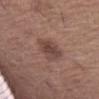image: 15 mm crop, total-body photography | anatomic site: the left thigh | patient: female, approximately 65 years of age | diameter: about 3.5 mm | automated lesion analysis: a lesion area of about 7.5 mm², an eccentricity of roughly 0.5, and a shape-asymmetry score of about 0.25 (0 = symmetric); a mean CIELAB color near L≈44 a*≈19 b*≈23, roughly 9 lightness units darker than nearby skin, and a normalized lesion–skin contrast near 7.5; border irregularity of about 2.5 on a 0–10 scale, a color-variation rating of about 3/10, and peripheral color asymmetry of about 1; lesion-presence confidence of about 100/100 | tile lighting: white-light.